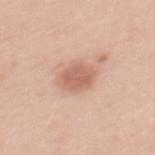Imaged during a routine full-body skin examination; the lesion was not biopsied and no histopathology is available. A 15 mm close-up tile from a total-body photography series done for melanoma screening. From the back. This is a white-light tile. The patient is a female aged around 40. Automated tile analysis of the lesion measured a mean CIELAB color near L≈62 a*≈22 b*≈30, a lesion–skin lightness drop of about 11, and a normalized border contrast of about 7. The software also gave border irregularity of about 1.5 on a 0–10 scale, internal color variation of about 2 on a 0–10 scale, and a peripheral color-asymmetry measure near 0.5. The analysis additionally found an automated nevus-likeness rating near 90 out of 100 and lesion-presence confidence of about 100/100.